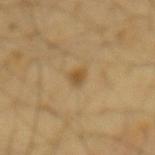Assessment:
This lesion was catalogued during total-body skin photography and was not selected for biopsy.
Background:
The subject is a male aged around 65. A 15 mm crop from a total-body photograph taken for skin-cancer surveillance. Located on the mid back.